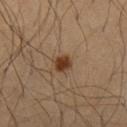Imaged during a routine full-body skin examination; the lesion was not biopsied and no histopathology is available.
A 15 mm close-up extracted from a 3D total-body photography capture.
The lesion's longest dimension is about 2 mm.
The lesion is located on the chest.
The subject is a male aged around 65.
Captured under cross-polarized illumination.
Automated image analysis of the tile measured an area of roughly 4 mm² and a shape-asymmetry score of about 0.15 (0 = symmetric). It also reported a lesion color around L≈35 a*≈19 b*≈30 in CIELAB and a lesion–skin lightness drop of about 12. And it measured lesion-presence confidence of about 100/100.